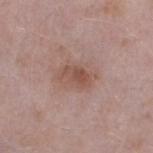The lesion was tiled from a total-body skin photograph and was not biopsied. Approximately 4 mm at its widest. This image is a 15 mm lesion crop taken from a total-body photograph. From the left lower leg. A male subject about 40 years old. Imaged with white-light lighting.